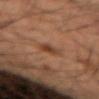| feature | finding |
|---|---|
| notes | no biopsy performed (imaged during a skin exam) |
| subject | male, approximately 35 years of age |
| automated lesion analysis | border irregularity of about 3.5 on a 0–10 scale, a within-lesion color-variation index near 4/10, and radial color variation of about 1.5; a nevus-likeness score of about 95/100 and a detector confidence of about 100 out of 100 that the crop contains a lesion |
| body site | the arm |
| imaging modality | ~15 mm crop, total-body skin-cancer survey |
| lighting | cross-polarized illumination |